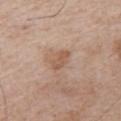Assessment:
No biopsy was performed on this lesion — it was imaged during a full skin examination and was not determined to be concerning.
Image and clinical context:
A close-up tile cropped from a whole-body skin photograph, about 15 mm across. The patient is a male aged approximately 65. The lesion is located on the front of the torso.